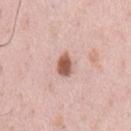workup: no biopsy performed (imaged during a skin exam); anatomic site: the chest; subject: male, aged 38–42; image: ~15 mm crop, total-body skin-cancer survey.